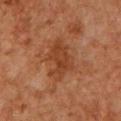Q: Is there a histopathology result?
A: total-body-photography surveillance lesion; no biopsy
Q: Where on the body is the lesion?
A: the upper back
Q: Who is the patient?
A: male, aged 58 to 62
Q: What kind of image is this?
A: ~15 mm crop, total-body skin-cancer survey